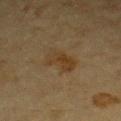<case>
  <biopsy_status>not biopsied; imaged during a skin examination</biopsy_status>
  <patient>
    <sex>male</sex>
    <age_approx>85+</age_approx>
  </patient>
  <image>
    <source>total-body photography crop</source>
    <field_of_view_mm>15</field_of_view_mm>
  </image>
  <lesion_size>
    <long_diameter_mm_approx>4.0</long_diameter_mm_approx>
  </lesion_size>
  <site>chest</site>
  <lighting>cross-polarized</lighting>
</case>